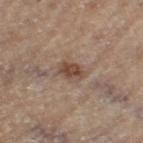follow-up — imaged on a skin check; not biopsied
acquisition — ~15 mm crop, total-body skin-cancer survey
illumination — cross-polarized
lesion diameter — about 3 mm
body site — the left thigh
subject — male, aged 83 or over